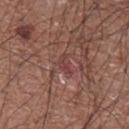Recorded during total-body skin imaging; not selected for excision or biopsy. From the chest. A male patient aged approximately 75. A 15 mm crop from a total-body photograph taken for skin-cancer surveillance.